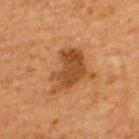This lesion was catalogued during total-body skin photography and was not selected for biopsy. The recorded lesion diameter is about 5.5 mm. A male patient, in their mid-60s. This is a cross-polarized tile. The lesion is located on the back. Cropped from a total-body skin-imaging series; the visible field is about 15 mm.